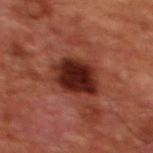Part of a total-body skin-imaging series; this lesion was reviewed on a skin check and was not flagged for biopsy. Located on the chest. A male patient, aged approximately 60. Cropped from a whole-body photographic skin survey; the tile spans about 15 mm. An algorithmic analysis of the crop reported an area of roughly 16 mm² and a symmetry-axis asymmetry near 0.15. The analysis additionally found an automated nevus-likeness rating near 65 out of 100 and a lesion-detection confidence of about 100/100. Imaged with cross-polarized lighting.